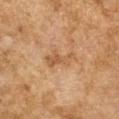Q: Is there a histopathology result?
A: no biopsy performed (imaged during a skin exam)
Q: What are the patient's age and sex?
A: female, roughly 60 years of age
Q: Lesion location?
A: the arm
Q: How large is the lesion?
A: about 4 mm
Q: How was the tile lit?
A: cross-polarized
Q: What is the imaging modality?
A: ~15 mm crop, total-body skin-cancer survey
Q: Automated lesion metrics?
A: a footprint of about 5.5 mm², a shape eccentricity near 0.85, and a symmetry-axis asymmetry near 0.4; a lesion color around L≈49 a*≈18 b*≈34 in CIELAB, about 7 CIELAB-L* units darker than the surrounding skin, and a normalized border contrast of about 5.5; a border-irregularity index near 5/10 and a within-lesion color-variation index near 2/10